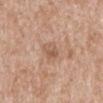Impression:
This lesion was catalogued during total-body skin photography and was not selected for biopsy.
Background:
The lesion is located on the mid back. A lesion tile, about 15 mm wide, cut from a 3D total-body photograph. The subject is a male aged around 65. Approximately 3 mm at its widest.